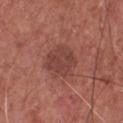| key | value |
|---|---|
| notes | total-body-photography surveillance lesion; no biopsy |
| acquisition | 15 mm crop, total-body photography |
| patient | male, aged 73–77 |
| body site | the chest |
| illumination | white-light illumination |